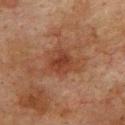workup = no biopsy performed (imaged during a skin exam) | illumination = cross-polarized illumination | lesion diameter = ≈5 mm | location = the upper back | patient = male, about 75 years old | image source = total-body-photography crop, ~15 mm field of view | automated metrics = a lesion color around L≈35 a*≈20 b*≈27 in CIELAB; an automated nevus-likeness rating near 25 out of 100.